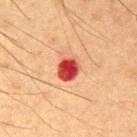{"biopsy_status": "not biopsied; imaged during a skin examination"}